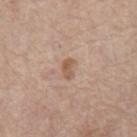notes=imaged on a skin check; not biopsied
tile lighting=white-light illumination
acquisition=total-body-photography crop, ~15 mm field of view
body site=the chest
diameter=≈2.5 mm
patient=male, aged 63 to 67